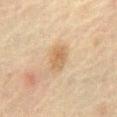No biopsy was performed on this lesion — it was imaged during a full skin examination and was not determined to be concerning. A male subject, aged around 70. A 15 mm close-up tile from a total-body photography series done for melanoma screening. The recorded lesion diameter is about 3.5 mm. On the abdomen.